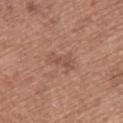| field | value |
|---|---|
| workup | catalogued during a skin exam; not biopsied |
| illumination | white-light |
| image | ~15 mm crop, total-body skin-cancer survey |
| patient | female, roughly 60 years of age |
| site | the upper back |